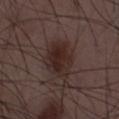Recorded during total-body skin imaging; not selected for excision or biopsy. The recorded lesion diameter is about 4.5 mm. Captured under white-light illumination. A male subject, approximately 50 years of age. A lesion tile, about 15 mm wide, cut from a 3D total-body photograph. Automated tile analysis of the lesion measured an eccentricity of roughly 0.6 and a symmetry-axis asymmetry near 0.2. And it measured an average lesion color of about L≈25 a*≈15 b*≈17 (CIELAB), about 8 CIELAB-L* units darker than the surrounding skin, and a lesion-to-skin contrast of about 9 (normalized; higher = more distinct). It also reported a classifier nevus-likeness of about 90/100.